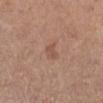Case summary:
* notes · imaged on a skin check; not biopsied
* imaging modality · ~15 mm crop, total-body skin-cancer survey
* patient · female, roughly 55 years of age
* TBP lesion metrics · an average lesion color of about L≈52 a*≈20 b*≈28 (CIELAB) and about 7 CIELAB-L* units darker than the surrounding skin; border irregularity of about 3 on a 0–10 scale and internal color variation of about 1 on a 0–10 scale; a lesion-detection confidence of about 100/100
* body site · the right lower leg